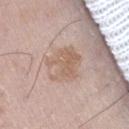* workup — no biopsy performed (imaged during a skin exam)
* patient — male, aged around 65
* location — the lower back
* imaging modality — total-body-photography crop, ~15 mm field of view
* lesion diameter — ≈5 mm